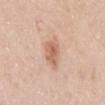follow-up: total-body-photography surveillance lesion; no biopsy | image: 15 mm crop, total-body photography | body site: the mid back | patient: male, approximately 40 years of age.